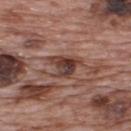follow-up = catalogued during a skin exam; not biopsied | TBP lesion metrics = a lesion area of about 8 mm² and a symmetry-axis asymmetry near 0.4; a within-lesion color-variation index near 9.5/10 and peripheral color asymmetry of about 3; an automated nevus-likeness rating near 0 out of 100 and a lesion-detection confidence of about 95/100 | imaging modality = ~15 mm tile from a whole-body skin photo | diameter = about 4 mm | anatomic site = the upper back | subject = male, about 70 years old | lighting = white-light.